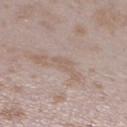Clinical impression: The lesion was photographed on a routine skin check and not biopsied; there is no pathology result. Image and clinical context: A female subject in their mid- to late 20s. About 2.5 mm across. Captured under white-light illumination. A region of skin cropped from a whole-body photographic capture, roughly 15 mm wide. An algorithmic analysis of the crop reported an area of roughly 3 mm², an eccentricity of roughly 0.85, and two-axis asymmetry of about 0.3. The software also gave border irregularity of about 3 on a 0–10 scale and radial color variation of about 0. The analysis additionally found a detector confidence of about 75 out of 100 that the crop contains a lesion. The lesion is on the left lower leg.